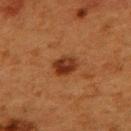This lesion was catalogued during total-body skin photography and was not selected for biopsy. About 3 mm across. On the upper back. The tile uses cross-polarized illumination. A 15 mm close-up extracted from a 3D total-body photography capture. A female patient, aged around 40.